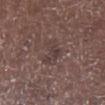Imaged during a routine full-body skin examination; the lesion was not biopsied and no histopathology is available. The total-body-photography lesion software estimated a lesion color around L≈39 a*≈15 b*≈18 in CIELAB, roughly 6 lightness units darker than nearby skin, and a normalized border contrast of about 5. The software also gave a border-irregularity rating of about 5.5/10, a within-lesion color-variation index near 3/10, and peripheral color asymmetry of about 1. The software also gave a classifier nevus-likeness of about 0/100 and a lesion-detection confidence of about 85/100. The subject is a male aged around 70. A 15 mm close-up extracted from a 3D total-body photography capture. From the left lower leg. This is a white-light tile. Longest diameter approximately 4 mm.